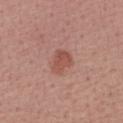Findings:
* follow-up: total-body-photography surveillance lesion; no biopsy
* acquisition: total-body-photography crop, ~15 mm field of view
* location: the right forearm
* lesion size: ≈3.5 mm
* tile lighting: white-light
* patient: female, in their mid-50s
* automated lesion analysis: a border-irregularity rating of about 3.5/10, internal color variation of about 3 on a 0–10 scale, and peripheral color asymmetry of about 1